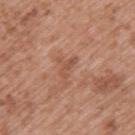Assessment: Recorded during total-body skin imaging; not selected for excision or biopsy. Clinical summary: A male patient aged 48 to 52. Approximately 2.5 mm at its widest. Automated image analysis of the tile measured an area of roughly 2 mm². The software also gave a lesion–skin lightness drop of about 7 and a normalized lesion–skin contrast near 5. It also reported a border-irregularity index near 5/10 and a color-variation rating of about 0/10. The lesion is on the upper back. Cropped from a total-body skin-imaging series; the visible field is about 15 mm.